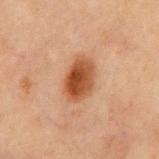No biopsy was performed on this lesion — it was imaged during a full skin examination and was not determined to be concerning.
The lesion's longest dimension is about 4.5 mm.
The lesion is on the chest.
A 15 mm close-up extracted from a 3D total-body photography capture.
A male patient aged 68–72.
An algorithmic analysis of the crop reported an outline eccentricity of about 0.8 (0 = round, 1 = elongated) and a shape-asymmetry score of about 0.2 (0 = symmetric). The analysis additionally found a classifier nevus-likeness of about 100/100.